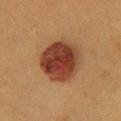Assessment:
No biopsy was performed on this lesion — it was imaged during a full skin examination and was not determined to be concerning.
Context:
A 15 mm close-up extracted from a 3D total-body photography capture. The lesion is on the chest. A female subject, in their 40s. Imaged with cross-polarized lighting. The lesion's longest dimension is about 5 mm. The lesion-visualizer software estimated a lesion color around L≈34 a*≈23 b*≈28 in CIELAB and about 14 CIELAB-L* units darker than the surrounding skin. It also reported a classifier nevus-likeness of about 100/100 and a lesion-detection confidence of about 100/100.